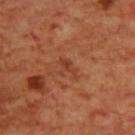A 15 mm close-up extracted from a 3D total-body photography capture. Located on the back. Imaged with cross-polarized lighting. About 3 mm across. A male patient about 70 years old.